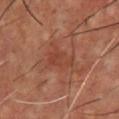biopsy_status: not biopsied; imaged during a skin examination
patient:
  sex: male
  age_approx: 55
site: chest
image:
  source: total-body photography crop
  field_of_view_mm: 15